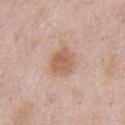Findings:
* workup — no biopsy performed (imaged during a skin exam)
* lighting — white-light illumination
* subject — male, in their mid-50s
* image — ~15 mm crop, total-body skin-cancer survey
* body site — the abdomen
* automated lesion analysis — an average lesion color of about L≈60 a*≈21 b*≈30 (CIELAB) and about 10 CIELAB-L* units darker than the surrounding skin